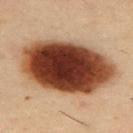Notes:
– notes · no biopsy performed (imaged during a skin exam)
– image · ~15 mm tile from a whole-body skin photo
– anatomic site · the back
– subject · male, aged approximately 40
– lesion size · ~11.5 mm (longest diameter)
– lighting · cross-polarized illumination